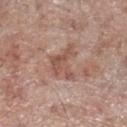Clinical impression: The lesion was tiled from a total-body skin photograph and was not biopsied. Acquisition and patient details: A male patient about 70 years old. The tile uses white-light illumination. Automated image analysis of the tile measured an area of roughly 8 mm², an eccentricity of roughly 0.65, and a symmetry-axis asymmetry near 0.6. It also reported a mean CIELAB color near L≈53 a*≈21 b*≈26 and a normalized border contrast of about 6.5. It also reported a classifier nevus-likeness of about 0/100 and a lesion-detection confidence of about 100/100. A 15 mm crop from a total-body photograph taken for skin-cancer surveillance. The lesion is located on the right lower leg.